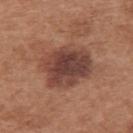Notes:
* biopsy status · total-body-photography surveillance lesion; no biopsy
* patient · female, roughly 40 years of age
* lesion diameter · ~6 mm (longest diameter)
* image · 15 mm crop, total-body photography
* illumination · white-light
* site · the upper back